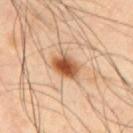This lesion was catalogued during total-body skin photography and was not selected for biopsy. Captured under cross-polarized illumination. A male subject, aged around 50. This image is a 15 mm lesion crop taken from a total-body photograph. On the upper back. Longest diameter approximately 4 mm.